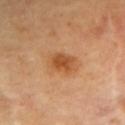The lesion was tiled from a total-body skin photograph and was not biopsied.
Approximately 4 mm at its widest.
The subject is a female roughly 60 years of age.
A close-up tile cropped from a whole-body skin photograph, about 15 mm across.
On the upper back.